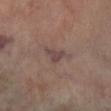Q: Was this lesion biopsied?
A: total-body-photography surveillance lesion; no biopsy
Q: What lighting was used for the tile?
A: cross-polarized
Q: What is the anatomic site?
A: the right lower leg
Q: What did automated image analysis measure?
A: a lesion area of about 3.5 mm² and a shape-asymmetry score of about 0.5 (0 = symmetric); about 7 CIELAB-L* units darker than the surrounding skin and a normalized border contrast of about 7; a border-irregularity rating of about 4.5/10, internal color variation of about 1 on a 0–10 scale, and radial color variation of about 0; a classifier nevus-likeness of about 0/100 and lesion-presence confidence of about 70/100
Q: What are the patient's age and sex?
A: male, roughly 70 years of age
Q: What kind of image is this?
A: total-body-photography crop, ~15 mm field of view